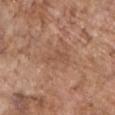Notes:
• follow-up: total-body-photography surveillance lesion; no biopsy
• imaging modality: ~15 mm crop, total-body skin-cancer survey
• location: the chest
• subject: male, about 70 years old
• lesion diameter: about 3.5 mm